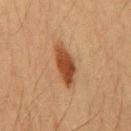{
  "biopsy_status": "not biopsied; imaged during a skin examination",
  "site": "front of the torso",
  "image": {
    "source": "total-body photography crop",
    "field_of_view_mm": 15
  },
  "lesion_size": {
    "long_diameter_mm_approx": 6.0
  },
  "patient": {
    "sex": "male",
    "age_approx": 65
  },
  "lighting": "cross-polarized"
}